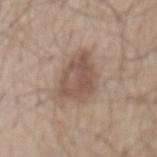Impression:
The lesion was tiled from a total-body skin photograph and was not biopsied.
Background:
The lesion is on the mid back. A region of skin cropped from a whole-body photographic capture, roughly 15 mm wide. This is a white-light tile. The patient is a male aged 48–52. Measured at roughly 5.5 mm in maximum diameter.